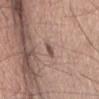follow-up: imaged on a skin check; not biopsied
patient: male, roughly 50 years of age
size: ≈2.5 mm
TBP lesion metrics: an automated nevus-likeness rating near 0 out of 100 and a detector confidence of about 100 out of 100 that the crop contains a lesion
location: the head or neck
lighting: white-light illumination
acquisition: ~15 mm crop, total-body skin-cancer survey